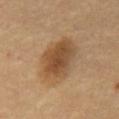biopsy_status: not biopsied; imaged during a skin examination
lighting: cross-polarized
image:
  source: total-body photography crop
  field_of_view_mm: 15
patient:
  sex: male
  age_approx: 55
automated_metrics:
  area_mm2_approx: 17.0
  eccentricity: 0.75
  shape_asymmetry: 0.15
  cielab_L: 45
  cielab_a: 17
  cielab_b: 33
  vs_skin_darker_L: 11.0
  vs_skin_contrast_norm: 8.5
  nevus_likeness_0_100: 100
  lesion_detection_confidence_0_100: 100
site: mid back